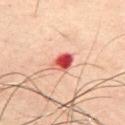| field | value |
|---|---|
| workup | imaged on a skin check; not biopsied |
| body site | the chest |
| automated metrics | an area of roughly 5 mm² and an outline eccentricity of about 0.7 (0 = round, 1 = elongated); a nevus-likeness score of about 0/100 and lesion-presence confidence of about 100/100 |
| image source | 15 mm crop, total-body photography |
| subject | male, roughly 50 years of age |
| lesion diameter | ~3 mm (longest diameter) |